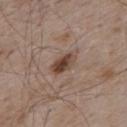Q: Was a biopsy performed?
A: catalogued during a skin exam; not biopsied
Q: What lighting was used for the tile?
A: white-light
Q: Who is the patient?
A: male, aged 53 to 57
Q: How was this image acquired?
A: ~15 mm crop, total-body skin-cancer survey
Q: Where on the body is the lesion?
A: the upper back
Q: What is the lesion's diameter?
A: ≈3.5 mm